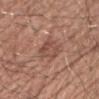imaging modality: total-body-photography crop, ~15 mm field of view | site: the chest | illumination: white-light illumination | TBP lesion metrics: a lesion area of about 7 mm² and an eccentricity of roughly 0.6; an automated nevus-likeness rating near 5 out of 100 and a lesion-detection confidence of about 100/100 | lesion size: ≈3.5 mm | patient: male, aged 53 to 57.